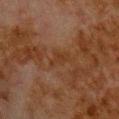| key | value |
|---|---|
| notes | no biopsy performed (imaged during a skin exam) |
| imaging modality | ~15 mm crop, total-body skin-cancer survey |
| image-analysis metrics | a lesion color around L≈27 a*≈17 b*≈26 in CIELAB, about 3 CIELAB-L* units darker than the surrounding skin, and a lesion-to-skin contrast of about 5 (normalized; higher = more distinct); internal color variation of about 0.5 on a 0–10 scale; an automated nevus-likeness rating near 0 out of 100 and a lesion-detection confidence of about 100/100 |
| subject | male, about 80 years old |
| lighting | cross-polarized |
| anatomic site | the back |